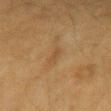Recorded during total-body skin imaging; not selected for excision or biopsy. A female patient, aged 53 to 57. A 15 mm close-up extracted from a 3D total-body photography capture. On the right forearm.